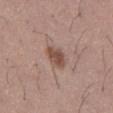Impression:
The lesion was tiled from a total-body skin photograph and was not biopsied.
Image and clinical context:
A male subject aged 43–47. A 15 mm close-up extracted from a 3D total-body photography capture. The lesion is located on the front of the torso.